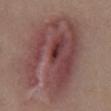Impression:
Part of a total-body skin-imaging series; this lesion was reviewed on a skin check and was not flagged for biopsy.
Acquisition and patient details:
This image is a 15 mm lesion crop taken from a total-body photograph. A female patient in their mid-50s. On the abdomen.